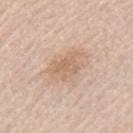<lesion>
<biopsy_status>not biopsied; imaged during a skin examination</biopsy_status>
<image>
  <source>total-body photography crop</source>
  <field_of_view_mm>15</field_of_view_mm>
</image>
<patient>
  <sex>male</sex>
  <age_approx>65</age_approx>
</patient>
<site>left upper arm</site>
<lighting>white-light</lighting>
<lesion_size>
  <long_diameter_mm_approx>4.5</long_diameter_mm_approx>
</lesion_size>
</lesion>